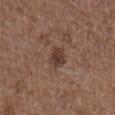Captured during whole-body skin photography for melanoma surveillance; the lesion was not biopsied. This is a white-light tile. Cropped from a total-body skin-imaging series; the visible field is about 15 mm. The patient is a male aged around 50. Longest diameter approximately 2.5 mm. Located on the left upper arm.